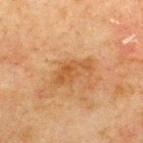notes=catalogued during a skin exam; not biopsied